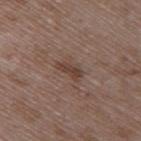Impression: No biopsy was performed on this lesion — it was imaged during a full skin examination and was not determined to be concerning. Image and clinical context: The patient is a male roughly 50 years of age. From the arm. Longest diameter approximately 3 mm. The tile uses white-light illumination. A lesion tile, about 15 mm wide, cut from a 3D total-body photograph.